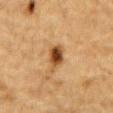<record>
  <lesion_size>
    <long_diameter_mm_approx>2.5</long_diameter_mm_approx>
  </lesion_size>
  <lighting>cross-polarized</lighting>
  <image>
    <source>total-body photography crop</source>
    <field_of_view_mm>15</field_of_view_mm>
  </image>
  <site>mid back</site>
  <patient>
    <sex>male</sex>
    <age_approx>85+</age_approx>
  </patient>
</record>